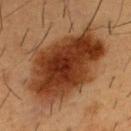- workup · total-body-photography surveillance lesion; no biopsy
- site · the front of the torso
- imaging modality · ~15 mm crop, total-body skin-cancer survey
- tile lighting · cross-polarized illumination
- patient · male, about 55 years old
- automated metrics · a border-irregularity index near 3/10 and peripheral color asymmetry of about 2.5; a classifier nevus-likeness of about 100/100 and lesion-presence confidence of about 100/100